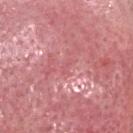This lesion was catalogued during total-body skin photography and was not selected for biopsy. The tile uses white-light illumination. Located on the head or neck. Cropped from a total-body skin-imaging series; the visible field is about 15 mm. The subject is a female aged 58 to 62. Longest diameter approximately 2 mm. The lesion-visualizer software estimated a lesion color around L≈58 a*≈32 b*≈21 in CIELAB and roughly 5 lightness units darker than nearby skin. The analysis additionally found a border-irregularity index near 3.5/10, internal color variation of about 0 on a 0–10 scale, and peripheral color asymmetry of about 0.